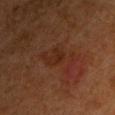Clinical impression:
Recorded during total-body skin imaging; not selected for excision or biopsy.
Image and clinical context:
The recorded lesion diameter is about 6 mm. A male subject aged around 65. An algorithmic analysis of the crop reported a footprint of about 11 mm², an eccentricity of roughly 0.9, and a shape-asymmetry score of about 0.45 (0 = symmetric). The software also gave a border-irregularity rating of about 7/10, internal color variation of about 2 on a 0–10 scale, and radial color variation of about 0.5. The lesion is located on the front of the torso. The tile uses cross-polarized illumination. A roughly 15 mm field-of-view crop from a total-body skin photograph.